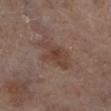Findings:
* subject · female, aged 58 to 62
* lesion size · about 4.5 mm
* image source · ~15 mm crop, total-body skin-cancer survey
* body site · the left lower leg
* illumination · cross-polarized